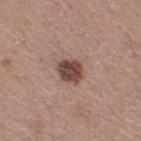Q: Was this lesion biopsied?
A: catalogued during a skin exam; not biopsied
Q: Lesion location?
A: the right thigh
Q: How was this image acquired?
A: ~15 mm crop, total-body skin-cancer survey
Q: What is the lesion's diameter?
A: ~3.5 mm (longest diameter)
Q: Who is the patient?
A: female, roughly 55 years of age
Q: How was the tile lit?
A: white-light illumination